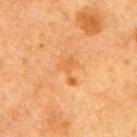Captured during whole-body skin photography for melanoma surveillance; the lesion was not biopsied.
The patient is a female aged approximately 40.
From the right upper arm.
Cropped from a total-body skin-imaging series; the visible field is about 15 mm.